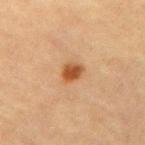Case summary:
• biopsy status — total-body-photography surveillance lesion; no biopsy
• tile lighting — cross-polarized
• location — the right upper arm
• patient — female, approximately 65 years of age
• imaging modality — 15 mm crop, total-body photography
• lesion diameter — ~2.5 mm (longest diameter)